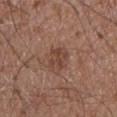Findings:
– notes — no biopsy performed (imaged during a skin exam)
– illumination — white-light illumination
– lesion size — ~3 mm (longest diameter)
– body site — the front of the torso
– image source — ~15 mm crop, total-body skin-cancer survey
– subject — male, approximately 75 years of age